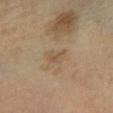This lesion was catalogued during total-body skin photography and was not selected for biopsy. A male subject, roughly 65 years of age. Captured under cross-polarized illumination. A 15 mm close-up extracted from a 3D total-body photography capture. From the left lower leg.